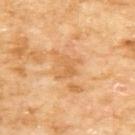Q: Patient demographics?
A: female, aged 58 to 62
Q: What kind of image is this?
A: total-body-photography crop, ~15 mm field of view
Q: What is the lesion's diameter?
A: ≈2.5 mm
Q: Lesion location?
A: the upper back